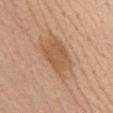Assessment: The lesion was tiled from a total-body skin photograph and was not biopsied. Background: Cropped from a total-body skin-imaging series; the visible field is about 15 mm. An algorithmic analysis of the crop reported about 9 CIELAB-L* units darker than the surrounding skin and a normalized border contrast of about 6.5. The analysis additionally found a lesion-detection confidence of about 100/100. This is a white-light tile. The lesion is located on the abdomen. About 6 mm across. The patient is a female roughly 60 years of age.